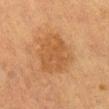Clinical impression: Part of a total-body skin-imaging series; this lesion was reviewed on a skin check and was not flagged for biopsy. Context: Automated tile analysis of the lesion measured a classifier nevus-likeness of about 25/100 and a lesion-detection confidence of about 100/100. The lesion is located on the abdomen. Cropped from a total-body skin-imaging series; the visible field is about 15 mm. The patient is a female about 55 years old. Imaged with cross-polarized lighting.